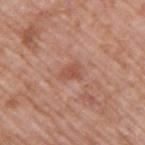Captured during whole-body skin photography for melanoma surveillance; the lesion was not biopsied. A male subject aged 68–72. The lesion-visualizer software estimated radial color variation of about 0.5. The lesion is on the right upper arm. A lesion tile, about 15 mm wide, cut from a 3D total-body photograph.